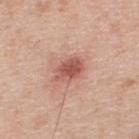Assessment:
The lesion was tiled from a total-body skin photograph and was not biopsied.
Context:
Captured under white-light illumination. Located on the back. Measured at roughly 4 mm in maximum diameter. A roughly 15 mm field-of-view crop from a total-body skin photograph. A male patient, aged 38 to 42.